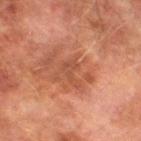{"patient": {"sex": "male", "age_approx": 75}, "site": "left lower leg", "lesion_size": {"long_diameter_mm_approx": 3.0}, "image": {"source": "total-body photography crop", "field_of_view_mm": 15}}